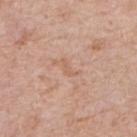{
  "biopsy_status": "not biopsied; imaged during a skin examination",
  "patient": {
    "sex": "female",
    "age_approx": 60
  },
  "lesion_size": {
    "long_diameter_mm_approx": 2.5
  },
  "image": {
    "source": "total-body photography crop",
    "field_of_view_mm": 15
  },
  "site": "right forearm"
}